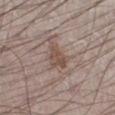Q: Was a biopsy performed?
A: catalogued during a skin exam; not biopsied
Q: Automated lesion metrics?
A: a detector confidence of about 100 out of 100 that the crop contains a lesion
Q: What is the anatomic site?
A: the left lower leg
Q: How was this image acquired?
A: ~15 mm crop, total-body skin-cancer survey
Q: How was the tile lit?
A: white-light illumination
Q: Patient demographics?
A: male, approximately 50 years of age